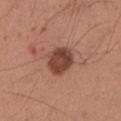| key | value |
|---|---|
| notes | catalogued during a skin exam; not biopsied |